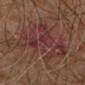This lesion was catalogued during total-body skin photography and was not selected for biopsy.
A lesion tile, about 15 mm wide, cut from a 3D total-body photograph.
The lesion's longest dimension is about 9.5 mm.
Captured under cross-polarized illumination.
The lesion-visualizer software estimated a footprint of about 36 mm² and two-axis asymmetry of about 0.4. The software also gave a normalized lesion–skin contrast near 6.5.
A male subject aged 58 to 62.
Located on the right leg.